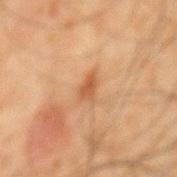<case>
<automated_metrics>
  <area_mm2_approx>3.5</area_mm2_approx>
  <border_irregularity_0_10>4.0</border_irregularity_0_10>
  <color_variation_0_10>2.0</color_variation_0_10>
  <peripheral_color_asymmetry>0.5</peripheral_color_asymmetry>
  <nevus_likeness_0_100>15</nevus_likeness_0_100>
</automated_metrics>
<site>mid back</site>
<lighting>cross-polarized</lighting>
<patient>
  <sex>male</sex>
  <age_approx>65</age_approx>
</patient>
<image>
  <source>total-body photography crop</source>
  <field_of_view_mm>15</field_of_view_mm>
</image>
</case>